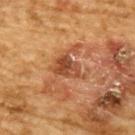Case summary:
- workup: no biopsy performed (imaged during a skin exam)
- site: the back
- automated metrics: a footprint of about 6.5 mm², an eccentricity of roughly 0.75, and a symmetry-axis asymmetry near 0.35; an average lesion color of about L≈41 a*≈23 b*≈32 (CIELAB) and about 10 CIELAB-L* units darker than the surrounding skin; a border-irregularity index near 4/10 and a peripheral color-asymmetry measure near 2; a nevus-likeness score of about 0/100 and a detector confidence of about 100 out of 100 that the crop contains a lesion
- acquisition: ~15 mm crop, total-body skin-cancer survey
- tile lighting: cross-polarized illumination
- subject: male, in their mid- to late 80s or older